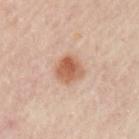Captured during whole-body skin photography for melanoma surveillance; the lesion was not biopsied. On the left upper arm. Cropped from a whole-body photographic skin survey; the tile spans about 15 mm. This is a cross-polarized tile. A patient aged 53–57. An algorithmic analysis of the crop reported a lesion area of about 7.5 mm², an outline eccentricity of about 0.45 (0 = round, 1 = elongated), and two-axis asymmetry of about 0.15. The analysis additionally found roughly 12 lightness units darker than nearby skin. It also reported an automated nevus-likeness rating near 100 out of 100 and lesion-presence confidence of about 100/100. Approximately 3.5 mm at its widest.